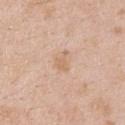body site=the upper back | lesion diameter=≈2.5 mm | image source=total-body-photography crop, ~15 mm field of view | image-analysis metrics=a lesion area of about 3 mm²; an average lesion color of about L≈65 a*≈18 b*≈32 (CIELAB) and a normalized border contrast of about 5.5 | subject=male, about 25 years old | tile lighting=white-light.